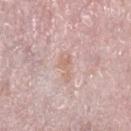Impression:
The lesion was tiled from a total-body skin photograph and was not biopsied.
Clinical summary:
A female patient, aged approximately 40. The tile uses white-light illumination. From the right lower leg. The lesion-visualizer software estimated an area of roughly 4 mm² and a shape-asymmetry score of about 0.35 (0 = symmetric). The software also gave a mean CIELAB color near L≈65 a*≈19 b*≈25, roughly 6 lightness units darker than nearby skin, and a lesion-to-skin contrast of about 5.5 (normalized; higher = more distinct). A roughly 15 mm field-of-view crop from a total-body skin photograph.